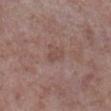Findings:
- notes: no biopsy performed (imaged during a skin exam)
- size: ≈2.5 mm
- location: the right lower leg
- subject: male, about 70 years old
- acquisition: 15 mm crop, total-body photography